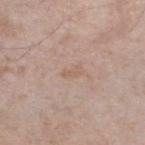Recorded during total-body skin imaging; not selected for excision or biopsy.
Approximately 2.5 mm at its widest.
The tile uses white-light illumination.
A male subject aged around 60.
The total-body-photography lesion software estimated an area of roughly 2.5 mm², a shape eccentricity near 0.9, and a shape-asymmetry score of about 0.35 (0 = symmetric). And it measured an average lesion color of about L≈60 a*≈16 b*≈27 (CIELAB), roughly 5 lightness units darker than nearby skin, and a normalized border contrast of about 4.5. The analysis additionally found a border-irregularity rating of about 3.5/10, internal color variation of about 0 on a 0–10 scale, and radial color variation of about 0. And it measured lesion-presence confidence of about 100/100.
This image is a 15 mm lesion crop taken from a total-body photograph.
On the left lower leg.